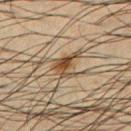biopsy status: total-body-photography surveillance lesion; no biopsy | diameter: ~3.5 mm (longest diameter) | body site: the chest | image source: total-body-photography crop, ~15 mm field of view | tile lighting: cross-polarized illumination | subject: male, about 35 years old.